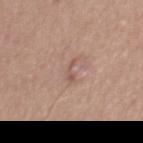Case summary:
• follow-up · imaged on a skin check; not biopsied
• site · the leg
• lesion size · about 2.5 mm
• automated lesion analysis · a nevus-likeness score of about 0/100 and lesion-presence confidence of about 100/100
• subject · female, about 55 years old
• imaging modality · 15 mm crop, total-body photography